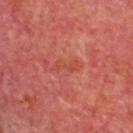The lesion was tiled from a total-body skin photograph and was not biopsied. The lesion's longest dimension is about 3 mm. Captured under cross-polarized illumination. An algorithmic analysis of the crop reported about 5 CIELAB-L* units darker than the surrounding skin. And it measured a nevus-likeness score of about 0/100 and a detector confidence of about 100 out of 100 that the crop contains a lesion. A male subject, about 65 years old. On the head or neck. A close-up tile cropped from a whole-body skin photograph, about 15 mm across.